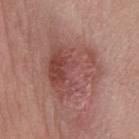biopsy status: catalogued during a skin exam; not biopsied
subject: male, aged 53 to 57
lesion size: ~7.5 mm (longest diameter)
anatomic site: the chest
image source: 15 mm crop, total-body photography
automated metrics: an area of roughly 32 mm²; an automated nevus-likeness rating near 25 out of 100 and a detector confidence of about 100 out of 100 that the crop contains a lesion